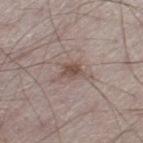Recorded during total-body skin imaging; not selected for excision or biopsy. A close-up tile cropped from a whole-body skin photograph, about 15 mm across. The lesion-visualizer software estimated a footprint of about 3.5 mm². The software also gave a lesion color around L≈49 a*≈16 b*≈22 in CIELAB, about 10 CIELAB-L* units darker than the surrounding skin, and a lesion-to-skin contrast of about 7.5 (normalized; higher = more distinct). The analysis additionally found a border-irregularity rating of about 4/10, internal color variation of about 2.5 on a 0–10 scale, and peripheral color asymmetry of about 1. The analysis additionally found an automated nevus-likeness rating near 30 out of 100 and a lesion-detection confidence of about 100/100. The subject is a male aged 48 to 52. The lesion's longest dimension is about 2.5 mm. From the left thigh. The tile uses white-light illumination.